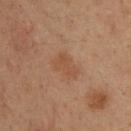Q: Was a biopsy performed?
A: no biopsy performed (imaged during a skin exam)
Q: How was this image acquired?
A: ~15 mm tile from a whole-body skin photo
Q: Lesion size?
A: about 3.5 mm
Q: Automated lesion metrics?
A: a mean CIELAB color near L≈45 a*≈19 b*≈30, about 6 CIELAB-L* units darker than the surrounding skin, and a lesion-to-skin contrast of about 5.5 (normalized; higher = more distinct); lesion-presence confidence of about 100/100
Q: Patient demographics?
A: male, in their mid-30s
Q: What lighting was used for the tile?
A: cross-polarized illumination
Q: Lesion location?
A: the upper back